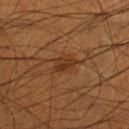{
  "image": {
    "source": "total-body photography crop",
    "field_of_view_mm": 15
  },
  "site": "right lower leg",
  "patient": {
    "sex": "male",
    "age_approx": 55
  },
  "lighting": "cross-polarized"
}